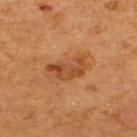The lesion is on the upper back. This is a cross-polarized tile. A region of skin cropped from a whole-body photographic capture, roughly 15 mm wide. A male patient about 65 years old. Measured at roughly 5 mm in maximum diameter.